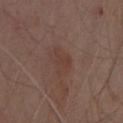Case summary:
• follow-up · catalogued during a skin exam; not biopsied
• automated metrics · about 5 CIELAB-L* units darker than the surrounding skin and a lesion-to-skin contrast of about 5 (normalized; higher = more distinct); a detector confidence of about 100 out of 100 that the crop contains a lesion
• size · about 3.5 mm
• site · the chest
• image · ~15 mm tile from a whole-body skin photo
• patient · male, aged around 70